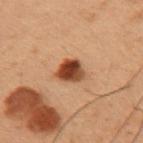{"patient": {"sex": "male", "age_approx": 55}, "lesion_size": {"long_diameter_mm_approx": 3.0}, "image": {"source": "total-body photography crop", "field_of_view_mm": 15}, "site": "left upper arm", "lighting": "cross-polarized"}